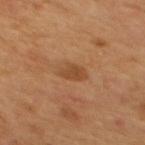The lesion was tiled from a total-body skin photograph and was not biopsied.
The total-body-photography lesion software estimated a shape eccentricity near 0.7 and a symmetry-axis asymmetry near 0.25. The analysis additionally found an average lesion color of about L≈42 a*≈20 b*≈33 (CIELAB) and about 7 CIELAB-L* units darker than the surrounding skin. The software also gave a border-irregularity rating of about 2.5/10, a within-lesion color-variation index near 2/10, and radial color variation of about 1. The analysis additionally found an automated nevus-likeness rating near 20 out of 100 and a detector confidence of about 100 out of 100 that the crop contains a lesion.
The patient is a male aged 53–57.
Longest diameter approximately 3 mm.
Located on the mid back.
This is a cross-polarized tile.
This image is a 15 mm lesion crop taken from a total-body photograph.